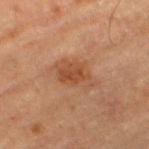The tile uses cross-polarized illumination. A 15 mm crop from a total-body photograph taken for skin-cancer surveillance. The patient is a male about 85 years old or older. Measured at roughly 4.5 mm in maximum diameter. On the left thigh.